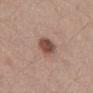No biopsy was performed on this lesion — it was imaged during a full skin examination and was not determined to be concerning. Imaged with white-light lighting. Longest diameter approximately 3 mm. A 15 mm close-up extracted from a 3D total-body photography capture. On the mid back. The subject is a male roughly 70 years of age.